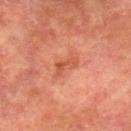Context:
The patient is a male approximately 75 years of age. The lesion is on the right lower leg. A 15 mm close-up extracted from a 3D total-body photography capture. The lesion-visualizer software estimated a lesion area of about 3.5 mm² and an outline eccentricity of about 0.9 (0 = round, 1 = elongated). The analysis additionally found an automated nevus-likeness rating near 0 out of 100 and lesion-presence confidence of about 100/100. The recorded lesion diameter is about 3.5 mm. Captured under cross-polarized illumination.